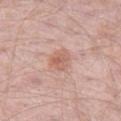Q: Was a biopsy performed?
A: imaged on a skin check; not biopsied
Q: Lesion location?
A: the left thigh
Q: How was the tile lit?
A: white-light
Q: What are the patient's age and sex?
A: male, in their 50s
Q: What kind of image is this?
A: 15 mm crop, total-body photography